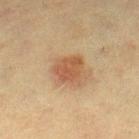Q: Is there a histopathology result?
A: catalogued during a skin exam; not biopsied
Q: Lesion location?
A: the right lower leg
Q: What are the patient's age and sex?
A: female, aged around 55
Q: How large is the lesion?
A: ~3 mm (longest diameter)
Q: How was the tile lit?
A: cross-polarized illumination
Q: What is the imaging modality?
A: 15 mm crop, total-body photography